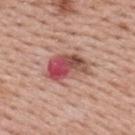Imaged during a routine full-body skin examination; the lesion was not biopsied and no histopathology is available.
The tile uses white-light illumination.
The lesion is on the upper back.
A male subject aged around 40.
About 5 mm across.
Cropped from a whole-body photographic skin survey; the tile spans about 15 mm.